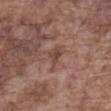The lesion was photographed on a routine skin check and not biopsied; there is no pathology result.
From the front of the torso.
A 15 mm crop from a total-body photograph taken for skin-cancer surveillance.
A male patient, aged 73 to 77.